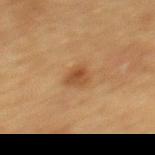Imaged during a routine full-body skin examination; the lesion was not biopsied and no histopathology is available. A 15 mm crop from a total-body photograph taken for skin-cancer surveillance. A female subject aged 68 to 72. On the upper back.